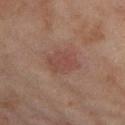Impression:
The lesion was tiled from a total-body skin photograph and was not biopsied.